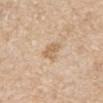Part of a total-body skin-imaging series; this lesion was reviewed on a skin check and was not flagged for biopsy. On the abdomen. The lesion's longest dimension is about 2.5 mm. A male subject in their 70s. The total-body-photography lesion software estimated a lesion area of about 5 mm², an outline eccentricity of about 0.5 (0 = round, 1 = elongated), and a shape-asymmetry score of about 0.3 (0 = symmetric). And it measured a lesion–skin lightness drop of about 9 and a normalized lesion–skin contrast near 6. It also reported border irregularity of about 3 on a 0–10 scale, internal color variation of about 2 on a 0–10 scale, and a peripheral color-asymmetry measure near 0.5. It also reported a nevus-likeness score of about 10/100 and lesion-presence confidence of about 100/100. A close-up tile cropped from a whole-body skin photograph, about 15 mm across. The tile uses white-light illumination.